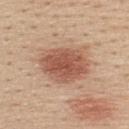Notes:
* biopsy status: catalogued during a skin exam; not biopsied
* acquisition: ~15 mm crop, total-body skin-cancer survey
* illumination: white-light illumination
* subject: female, in their mid- to late 40s
* lesion size: about 6 mm
* body site: the back